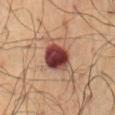| feature | finding |
|---|---|
| biopsy status | no biopsy performed (imaged during a skin exam) |
| tile lighting | cross-polarized illumination |
| TBP lesion metrics | a shape eccentricity near 0.55 and two-axis asymmetry of about 0.1; a mean CIELAB color near L≈36 a*≈24 b*≈21, about 19 CIELAB-L* units darker than the surrounding skin, and a normalized border contrast of about 15.5; a border-irregularity index near 1.5/10, a color-variation rating of about 6.5/10, and radial color variation of about 2 |
| image | total-body-photography crop, ~15 mm field of view |
| size | about 4 mm |
| subject | male, aged 58 to 62 |
| body site | the abdomen |